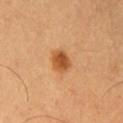Assessment:
Imaged during a routine full-body skin examination; the lesion was not biopsied and no histopathology is available.
Clinical summary:
From the left thigh. An algorithmic analysis of the crop reported a footprint of about 5.5 mm² and a shape eccentricity near 0.6. It also reported a mean CIELAB color near L≈53 a*≈26 b*≈42. And it measured a classifier nevus-likeness of about 100/100 and lesion-presence confidence of about 100/100. A 15 mm close-up extracted from a 3D total-body photography capture. A male subject in their 50s. This is a cross-polarized tile. The lesion's longest dimension is about 3 mm.